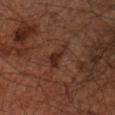Context:
The total-body-photography lesion software estimated an eccentricity of roughly 0.9 and a shape-asymmetry score of about 0.5 (0 = symmetric). It also reported an automated nevus-likeness rating near 5 out of 100 and a lesion-detection confidence of about 100/100. On the left arm. A male patient, aged approximately 65. Captured under cross-polarized illumination. A 15 mm close-up extracted from a 3D total-body photography capture.